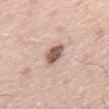<tbp_lesion>
  <biopsy_status>not biopsied; imaged during a skin examination</biopsy_status>
  <lighting>white-light</lighting>
  <patient>
    <sex>male</sex>
    <age_approx>75</age_approx>
  </patient>
  <site>mid back</site>
  <image>
    <source>total-body photography crop</source>
    <field_of_view_mm>15</field_of_view_mm>
  </image>
  <lesion_size>
    <long_diameter_mm_approx>3.5</long_diameter_mm_approx>
  </lesion_size>
</tbp_lesion>